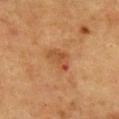Captured during whole-body skin photography for melanoma surveillance; the lesion was not biopsied. A lesion tile, about 15 mm wide, cut from a 3D total-body photograph. The lesion is on the chest. The patient is a female aged 53–57. This is a cross-polarized tile. The lesion's longest dimension is about 3.5 mm.